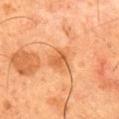Findings:
- subject — male, in their mid-60s
- imaging modality — total-body-photography crop, ~15 mm field of view
- site — the mid back
- lesion size — ≈3 mm